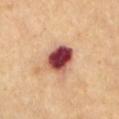Clinical impression: This lesion was catalogued during total-body skin photography and was not selected for biopsy. Image and clinical context: A close-up tile cropped from a whole-body skin photograph, about 15 mm across. The lesion's longest dimension is about 4 mm. Automated image analysis of the tile measured an area of roughly 11 mm² and a shape eccentricity near 0.55. The analysis additionally found a classifier nevus-likeness of about 0/100 and a lesion-detection confidence of about 100/100. The lesion is on the chest. The tile uses cross-polarized illumination. A female patient, roughly 70 years of age.